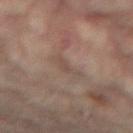<tbp_lesion>
  <biopsy_status>not biopsied; imaged during a skin examination</biopsy_status>
  <patient>
    <sex>female</sex>
    <age_approx>80</age_approx>
  </patient>
  <automated_metrics>
    <border_irregularity_0_10>5.0</border_irregularity_0_10>
    <color_variation_0_10>1.0</color_variation_0_10>
    <peripheral_color_asymmetry>0.0</peripheral_color_asymmetry>
  </automated_metrics>
  <image>
    <source>total-body photography crop</source>
    <field_of_view_mm>15</field_of_view_mm>
  </image>
  <lighting>cross-polarized</lighting>
  <site>left leg</site>
</tbp_lesion>